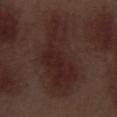workup: no biopsy performed (imaged during a skin exam) | acquisition: ~15 mm tile from a whole-body skin photo | tile lighting: white-light illumination | lesion size: ≈10.5 mm | location: the left thigh | image-analysis metrics: an area of roughly 34 mm², an outline eccentricity of about 0.9 (0 = round, 1 = elongated), and a symmetry-axis asymmetry near 0.3; an average lesion color of about L≈24 a*≈18 b*≈19 (CIELAB), about 5 CIELAB-L* units darker than the surrounding skin, and a lesion-to-skin contrast of about 6.5 (normalized; higher = more distinct); border irregularity of about 4.5 on a 0–10 scale, a color-variation rating of about 3/10, and a peripheral color-asymmetry measure near 1; an automated nevus-likeness rating near 0 out of 100 and a detector confidence of about 100 out of 100 that the crop contains a lesion | subject: male, aged approximately 70.